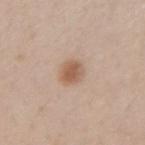{"biopsy_status": "not biopsied; imaged during a skin examination", "lesion_size": {"long_diameter_mm_approx": 3.0}, "lighting": "white-light", "patient": {"sex": "female", "age_approx": 25}, "image": {"source": "total-body photography crop", "field_of_view_mm": 15}, "site": "left forearm"}